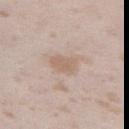Q: Was a biopsy performed?
A: imaged on a skin check; not biopsied
Q: How large is the lesion?
A: ~3 mm (longest diameter)
Q: Who is the patient?
A: female, aged around 25
Q: What is the anatomic site?
A: the left thigh
Q: How was this image acquired?
A: ~15 mm tile from a whole-body skin photo
Q: Automated lesion metrics?
A: a classifier nevus-likeness of about 0/100 and a detector confidence of about 100 out of 100 that the crop contains a lesion